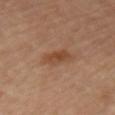Captured during whole-body skin photography for melanoma surveillance; the lesion was not biopsied.